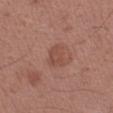Q: Was this lesion biopsied?
A: total-body-photography surveillance lesion; no biopsy